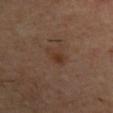• workup: no biopsy performed (imaged during a skin exam)
• illumination: cross-polarized
• site: the left upper arm
• patient: male, aged 53 to 57
• image source: ~15 mm tile from a whole-body skin photo